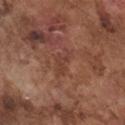image: ~15 mm tile from a whole-body skin photo
lighting: white-light illumination
automated metrics: an outline eccentricity of about 0.85 (0 = round, 1 = elongated) and two-axis asymmetry of about 0.45; a lesion color around L≈41 a*≈23 b*≈27 in CIELAB, a lesion–skin lightness drop of about 6, and a normalized border contrast of about 5
size: ~3.5 mm (longest diameter)
subject: male, aged 73 to 77
location: the chest